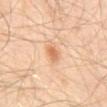workup = no biopsy performed (imaged during a skin exam) | anatomic site = the abdomen | lesion size = ~2.5 mm (longest diameter) | image source = 15 mm crop, total-body photography | patient = male, approximately 55 years of age | tile lighting = cross-polarized.